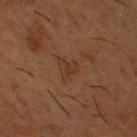Q: Was a biopsy performed?
A: no biopsy performed (imaged during a skin exam)
Q: What lighting was used for the tile?
A: cross-polarized illumination
Q: How large is the lesion?
A: ≈2.5 mm
Q: Who is the patient?
A: male, in their mid-60s
Q: What is the anatomic site?
A: the right thigh
Q: How was this image acquired?
A: 15 mm crop, total-body photography
Q: Automated lesion metrics?
A: an area of roughly 4.5 mm² and two-axis asymmetry of about 0.4; internal color variation of about 3 on a 0–10 scale; an automated nevus-likeness rating near 0 out of 100 and a lesion-detection confidence of about 100/100